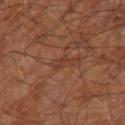Clinical impression: No biopsy was performed on this lesion — it was imaged during a full skin examination and was not determined to be concerning. Background: A lesion tile, about 15 mm wide, cut from a 3D total-body photograph. This is a cross-polarized tile. The subject is a male aged approximately 60. The lesion's longest dimension is about 2.5 mm. The lesion-visualizer software estimated an outline eccentricity of about 0.85 (0 = round, 1 = elongated) and a shape-asymmetry score of about 0.55 (0 = symmetric). The software also gave border irregularity of about 5.5 on a 0–10 scale, internal color variation of about 0 on a 0–10 scale, and peripheral color asymmetry of about 0. On the right leg.